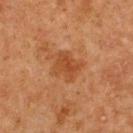Findings:
• biopsy status · imaged on a skin check; not biopsied
• patient · female, aged around 50
• illumination · cross-polarized illumination
• body site · the upper back
• lesion diameter · ~3 mm (longest diameter)
• acquisition · total-body-photography crop, ~15 mm field of view